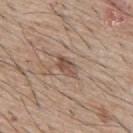Q: Was a biopsy performed?
A: imaged on a skin check; not biopsied
Q: What kind of image is this?
A: ~15 mm tile from a whole-body skin photo
Q: How large is the lesion?
A: about 3 mm
Q: What is the anatomic site?
A: the back
Q: Patient demographics?
A: male, aged 63–67
Q: Automated lesion metrics?
A: a symmetry-axis asymmetry near 0.3; border irregularity of about 3 on a 0–10 scale, a color-variation rating of about 4/10, and a peripheral color-asymmetry measure near 1.5